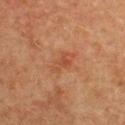Assessment: Part of a total-body skin-imaging series; this lesion was reviewed on a skin check and was not flagged for biopsy. Context: Measured at roughly 3.5 mm in maximum diameter. A female subject approximately 55 years of age. Captured under cross-polarized illumination. On the back. A close-up tile cropped from a whole-body skin photograph, about 15 mm across.